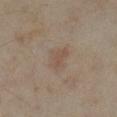<case>
  <biopsy_status>not biopsied; imaged during a skin examination</biopsy_status>
  <automated_metrics>
    <area_mm2_approx>5.5</area_mm2_approx>
    <eccentricity>0.65</eccentricity>
    <shape_asymmetry>0.35</shape_asymmetry>
  </automated_metrics>
  <lighting>cross-polarized</lighting>
  <site>right lower leg</site>
  <lesion_size>
    <long_diameter_mm_approx>3.0</long_diameter_mm_approx>
  </lesion_size>
  <image>
    <source>total-body photography crop</source>
    <field_of_view_mm>15</field_of_view_mm>
  </image>
  <patient>
    <sex>female</sex>
    <age_approx>35</age_approx>
  </patient>
</case>